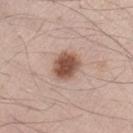lighting: white-light
lesion_size:
  long_diameter_mm_approx: 3.5
patient:
  sex: male
  age_approx: 45
automated_metrics:
  border_irregularity_0_10: 2.0
  peripheral_color_asymmetry: 1.0
site: left thigh
image:
  source: total-body photography crop
  field_of_view_mm: 15